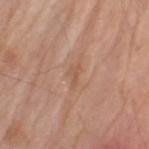acquisition: 15 mm crop, total-body photography
body site: the left upper arm
patient: male, about 80 years old